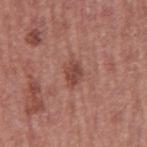Imaged during a routine full-body skin examination; the lesion was not biopsied and no histopathology is available. Automated tile analysis of the lesion measured a lesion-detection confidence of about 100/100. A 15 mm close-up extracted from a 3D total-body photography capture. The recorded lesion diameter is about 3 mm. Located on the left upper arm. The subject is a male about 45 years old.